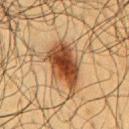biopsy status: catalogued during a skin exam; not biopsied | subject: male, in their 60s | site: the chest | image source: ~15 mm tile from a whole-body skin photo | tile lighting: cross-polarized.